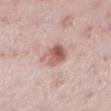follow-up: imaged on a skin check; not biopsied | subject: female, roughly 40 years of age | location: the left lower leg | image source: ~15 mm tile from a whole-body skin photo | tile lighting: white-light illumination | image-analysis metrics: a border-irregularity index near 2.5/10, a color-variation rating of about 7.5/10, and radial color variation of about 3; a nevus-likeness score of about 90/100 and a lesion-detection confidence of about 100/100.